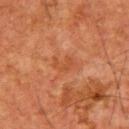Findings:
- biopsy status: imaged on a skin check; not biopsied
- image: 15 mm crop, total-body photography
- lesion size: ~3 mm (longest diameter)
- subject: male, approximately 65 years of age
- illumination: cross-polarized
- site: the upper back
- TBP lesion metrics: a border-irregularity rating of about 6/10, a color-variation rating of about 0/10, and peripheral color asymmetry of about 0; lesion-presence confidence of about 100/100